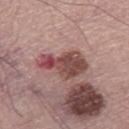No biopsy was performed on this lesion — it was imaged during a full skin examination and was not determined to be concerning. A male patient, aged around 70. The lesion is located on the leg. This image is a 15 mm lesion crop taken from a total-body photograph.